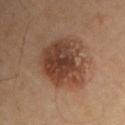{"biopsy_status": "not biopsied; imaged during a skin examination", "site": "left upper arm", "patient": {"sex": "male", "age_approx": 55}, "image": {"source": "total-body photography crop", "field_of_view_mm": 15}, "lighting": "cross-polarized", "automated_metrics": {"lesion_detection_confidence_0_100": 100}, "lesion_size": {"long_diameter_mm_approx": 7.0}}